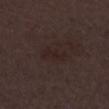  biopsy_status: not biopsied; imaged during a skin examination
  patient:
    sex: male
    age_approx: 50
  lighting: white-light
  image:
    source: total-body photography crop
    field_of_view_mm: 15
  lesion_size:
    long_diameter_mm_approx: 3.0